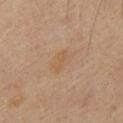No biopsy was performed on this lesion — it was imaged during a full skin examination and was not determined to be concerning.
On the abdomen.
Cropped from a whole-body photographic skin survey; the tile spans about 15 mm.
A male patient aged approximately 60.
This is a cross-polarized tile.
The lesion's longest dimension is about 3.5 mm.